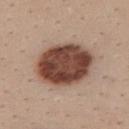The lesion was tiled from a total-body skin photograph and was not biopsied. Located on the upper back. Automated image analysis of the tile measured an eccentricity of roughly 0.6 and a shape-asymmetry score of about 0.1 (0 = symmetric). The software also gave a nevus-likeness score of about 75/100 and lesion-presence confidence of about 100/100. A 15 mm close-up extracted from a 3D total-body photography capture. A female subject, about 25 years old. The lesion's longest dimension is about 7 mm. This is a white-light tile.